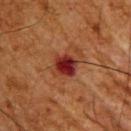notes=total-body-photography surveillance lesion; no biopsy | body site=the back | imaging modality=total-body-photography crop, ~15 mm field of view | patient=male, aged approximately 65.